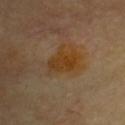Recorded during total-body skin imaging; not selected for excision or biopsy.
Approximately 4 mm at its widest.
This image is a 15 mm lesion crop taken from a total-body photograph.
This is a cross-polarized tile.
The subject is a male roughly 65 years of age.
The lesion is located on the chest.